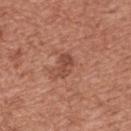follow-up: total-body-photography surveillance lesion; no biopsy | TBP lesion metrics: an area of roughly 4.5 mm², a shape eccentricity near 0.8, and a shape-asymmetry score of about 0.2 (0 = symmetric); an average lesion color of about L≈48 a*≈25 b*≈29 (CIELAB) and about 9 CIELAB-L* units darker than the surrounding skin | location: the front of the torso | tile lighting: white-light | patient: male, aged 43–47 | image: ~15 mm crop, total-body skin-cancer survey.